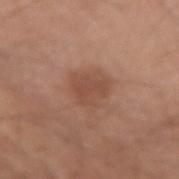The lesion was photographed on a routine skin check and not biopsied; there is no pathology result. The lesion's longest dimension is about 3.5 mm. Automated tile analysis of the lesion measured a nevus-likeness score of about 30/100 and lesion-presence confidence of about 100/100. Imaged with white-light lighting. A 15 mm close-up extracted from a 3D total-body photography capture. A male subject, about 70 years old. The lesion is located on the arm.